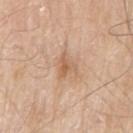Clinical impression: Part of a total-body skin-imaging series; this lesion was reviewed on a skin check and was not flagged for biopsy. Context: About 3 mm across. This is a white-light tile. This image is a 15 mm lesion crop taken from a total-body photograph. The subject is a male aged around 80. Located on the right upper arm.